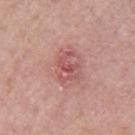follow-up: catalogued during a skin exam; not biopsied | patient: female, in their mid-60s | body site: the arm | lighting: white-light illumination | imaging modality: ~15 mm crop, total-body skin-cancer survey.